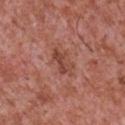subject: male, roughly 45 years of age; anatomic site: the chest; illumination: white-light illumination; imaging modality: ~15 mm tile from a whole-body skin photo; lesion size: about 4 mm.